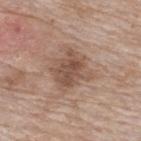Imaged during a routine full-body skin examination; the lesion was not biopsied and no histopathology is available. Approximately 4 mm at its widest. The subject is a female aged 73–77. A region of skin cropped from a whole-body photographic capture, roughly 15 mm wide. Captured under white-light illumination. On the upper back.